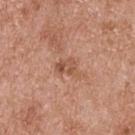Clinical impression: Captured during whole-body skin photography for melanoma surveillance; the lesion was not biopsied. Clinical summary: Located on the upper back. The lesion's longest dimension is about 3 mm. The total-body-photography lesion software estimated a lesion area of about 4.5 mm², a shape eccentricity near 0.6, and a shape-asymmetry score of about 0.3 (0 = symmetric). And it measured border irregularity of about 3 on a 0–10 scale and a within-lesion color-variation index near 4/10. The analysis additionally found an automated nevus-likeness rating near 0 out of 100 and a lesion-detection confidence of about 100/100. A close-up tile cropped from a whole-body skin photograph, about 15 mm across. Captured under white-light illumination. The subject is a male aged 53–57.